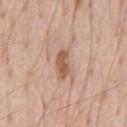A male subject, aged 53–57. This image is a 15 mm lesion crop taken from a total-body photograph. On the chest. The recorded lesion diameter is about 3.5 mm.